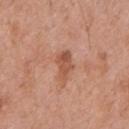Q: Was this lesion biopsied?
A: catalogued during a skin exam; not biopsied
Q: How was the tile lit?
A: white-light illumination
Q: Where on the body is the lesion?
A: the front of the torso
Q: How was this image acquired?
A: total-body-photography crop, ~15 mm field of view
Q: What did automated image analysis measure?
A: an area of roughly 6 mm² and an eccentricity of roughly 0.85; a lesion–skin lightness drop of about 10; a within-lesion color-variation index near 3/10; a nevus-likeness score of about 0/100
Q: Lesion size?
A: about 3.5 mm
Q: Patient demographics?
A: male, aged 68 to 72